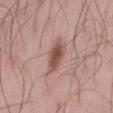Q: Was a biopsy performed?
A: catalogued during a skin exam; not biopsied
Q: What is the imaging modality?
A: 15 mm crop, total-body photography
Q: Lesion location?
A: the mid back
Q: Who is the patient?
A: male, approximately 45 years of age